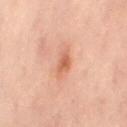biopsy status — imaged on a skin check; not biopsied
subject — female, roughly 55 years of age
lesion diameter — ~3.5 mm (longest diameter)
body site — the mid back
illumination — cross-polarized illumination
acquisition — 15 mm crop, total-body photography